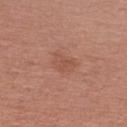Clinical impression: No biopsy was performed on this lesion — it was imaged during a full skin examination and was not determined to be concerning. Clinical summary: The lesion is located on the right upper arm. A close-up tile cropped from a whole-body skin photograph, about 15 mm across. A female subject, in their mid- to late 20s.